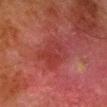Q: Was a biopsy performed?
A: no biopsy performed (imaged during a skin exam)
Q: What is the anatomic site?
A: the right lower leg
Q: Who is the patient?
A: male, roughly 80 years of age
Q: What kind of image is this?
A: ~15 mm crop, total-body skin-cancer survey